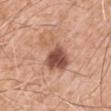Assessment: Imaged during a routine full-body skin examination; the lesion was not biopsied and no histopathology is available. Acquisition and patient details: A 15 mm close-up tile from a total-body photography series done for melanoma screening. Approximately 5 mm at its widest. From the right upper arm. The patient is a male approximately 60 years of age. Imaged with white-light lighting. Automated tile analysis of the lesion measured a mean CIELAB color near L≈54 a*≈23 b*≈30, about 14 CIELAB-L* units darker than the surrounding skin, and a normalized border contrast of about 9.